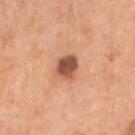Impression: Captured during whole-body skin photography for melanoma surveillance; the lesion was not biopsied. Image and clinical context: Imaged with white-light lighting. A roughly 15 mm field-of-view crop from a total-body skin photograph. Located on the right upper arm. A female patient, in their mid-50s.